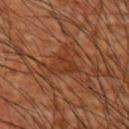Imaged during a routine full-body skin examination; the lesion was not biopsied and no histopathology is available.
This is a cross-polarized tile.
A 15 mm crop from a total-body photograph taken for skin-cancer surveillance.
The lesion is located on the right upper arm.
A male subject about 60 years old.
The lesion-visualizer software estimated a lesion area of about 7 mm², an eccentricity of roughly 0.6, and a shape-asymmetry score of about 0.6 (0 = symmetric). It also reported a within-lesion color-variation index near 3/10 and radial color variation of about 1. And it measured an automated nevus-likeness rating near 0 out of 100 and a detector confidence of about 85 out of 100 that the crop contains a lesion.
Approximately 4 mm at its widest.